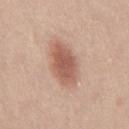No biopsy was performed on this lesion — it was imaged during a full skin examination and was not determined to be concerning. Located on the right thigh. A region of skin cropped from a whole-body photographic capture, roughly 15 mm wide. A female patient aged approximately 20. The lesion-visualizer software estimated a lesion area of about 13 mm², an outline eccentricity of about 0.85 (0 = round, 1 = elongated), and two-axis asymmetry of about 0.15. The analysis additionally found a lesion color around L≈57 a*≈22 b*≈28 in CIELAB, a lesion–skin lightness drop of about 12, and a normalized lesion–skin contrast near 8.